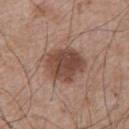Captured during whole-body skin photography for melanoma surveillance; the lesion was not biopsied. A region of skin cropped from a whole-body photographic capture, roughly 15 mm wide. Located on the left upper arm. The patient is a male approximately 65 years of age. The lesion's longest dimension is about 5 mm.